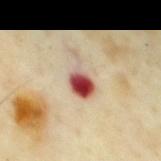Assessment: No biopsy was performed on this lesion — it was imaged during a full skin examination and was not determined to be concerning. Clinical summary: A 15 mm close-up extracted from a 3D total-body photography capture. This is a cross-polarized tile. Located on the chest. The subject is a male aged 63–67.